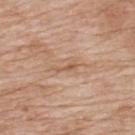The lesion was tiled from a total-body skin photograph and was not biopsied. A female patient about 65 years old. A lesion tile, about 15 mm wide, cut from a 3D total-body photograph. The lesion is on the upper back.